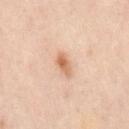This lesion was catalogued during total-body skin photography and was not selected for biopsy.
A male subject, aged 48–52.
Captured under cross-polarized illumination.
Located on the mid back.
A lesion tile, about 15 mm wide, cut from a 3D total-body photograph.
The lesion's longest dimension is about 3 mm.